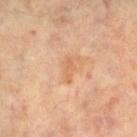biopsy_status: not biopsied; imaged during a skin examination
automated_metrics:
  cielab_L: 63
  cielab_a: 22
  cielab_b: 37
  vs_skin_contrast_norm: 5.0
  nevus_likeness_0_100: 0
  lesion_detection_confidence_0_100: 100
lighting: cross-polarized
image:
  source: total-body photography crop
  field_of_view_mm: 15
lesion_size:
  long_diameter_mm_approx: 3.0
site: left leg
patient:
  sex: female
  age_approx: 65